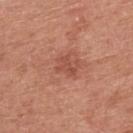Background:
This is a white-light tile. A roughly 15 mm field-of-view crop from a total-body skin photograph. On the arm. An algorithmic analysis of the crop reported a normalized lesion–skin contrast near 5.5. It also reported a detector confidence of about 100 out of 100 that the crop contains a lesion. A female subject, roughly 60 years of age. About 2.5 mm across.